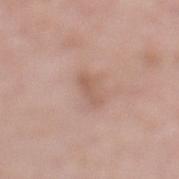The lesion was tiled from a total-body skin photograph and was not biopsied. The tile uses white-light illumination. On the left lower leg. The lesion-visualizer software estimated an average lesion color of about L≈58 a*≈19 b*≈27 (CIELAB). A 15 mm close-up extracted from a 3D total-body photography capture. A male patient, roughly 65 years of age.